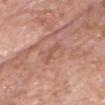Measured at roughly 3.5 mm in maximum diameter.
A close-up tile cropped from a whole-body skin photograph, about 15 mm across.
A male patient, aged 73 to 77.
On the head or neck.
Captured under white-light illumination.